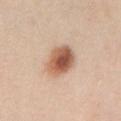The lesion was tiled from a total-body skin photograph and was not biopsied. A female subject, aged approximately 30. A close-up tile cropped from a whole-body skin photograph, about 15 mm across. On the front of the torso.